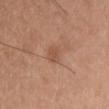<lesion>
  <biopsy_status>not biopsied; imaged during a skin examination</biopsy_status>
  <site>chest</site>
  <image>
    <source>total-body photography crop</source>
    <field_of_view_mm>15</field_of_view_mm>
  </image>
  <lighting>white-light</lighting>
  <automated_metrics>
    <cielab_L>52</cielab_L>
    <cielab_a>22</cielab_a>
    <cielab_b>31</cielab_b>
    <vs_skin_darker_L>7.0</vs_skin_darker_L>
    <vs_skin_contrast_norm>5.5</vs_skin_contrast_norm>
    <border_irregularity_0_10>2.5</border_irregularity_0_10>
    <color_variation_0_10>1.5</color_variation_0_10>
    <peripheral_color_asymmetry>0.5</peripheral_color_asymmetry>
    <nevus_likeness_0_100>0</nevus_likeness_0_100>
  </automated_metrics>
  <lesion_size>
    <long_diameter_mm_approx>3.0</long_diameter_mm_approx>
  </lesion_size>
  <patient>
    <sex>male</sex>
    <age_approx>35</age_approx>
  </patient>
</lesion>